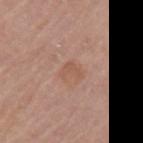  biopsy_status: not biopsied; imaged during a skin examination
  site: left upper arm
  patient:
    sex: female
    age_approx: 65
  lighting: white-light
  image:
    source: total-body photography crop
    field_of_view_mm: 15